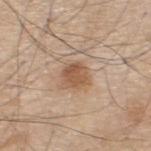No biopsy was performed on this lesion — it was imaged during a full skin examination and was not determined to be concerning. A 15 mm crop from a total-body photograph taken for skin-cancer surveillance. The patient is a male aged around 70. Imaged with white-light lighting. The lesion is on the back. The lesion-visualizer software estimated an area of roughly 6.5 mm², an outline eccentricity of about 0.35 (0 = round, 1 = elongated), and a shape-asymmetry score of about 0.2 (0 = symmetric). The software also gave an average lesion color of about L≈55 a*≈19 b*≈32 (CIELAB), roughly 11 lightness units darker than nearby skin, and a lesion-to-skin contrast of about 7.5 (normalized; higher = more distinct).